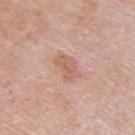Imaged during a routine full-body skin examination; the lesion was not biopsied and no histopathology is available. A male patient in their 60s. The tile uses white-light illumination. Automated tile analysis of the lesion measured a mean CIELAB color near L≈61 a*≈22 b*≈28, roughly 8 lightness units darker than nearby skin, and a normalized lesion–skin contrast near 5.5. The lesion is located on the left upper arm. A 15 mm close-up tile from a total-body photography series done for melanoma screening.